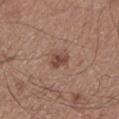Findings:
– biopsy status · no biopsy performed (imaged during a skin exam)
– patient · male, aged around 50
– illumination · white-light
– diameter · ≈2.5 mm
– image · ~15 mm crop, total-body skin-cancer survey
– location · the left lower leg